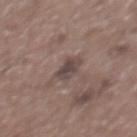Recorded during total-body skin imaging; not selected for excision or biopsy.
On the upper back.
Imaged with white-light lighting.
A male patient aged approximately 60.
A 15 mm close-up tile from a total-body photography series done for melanoma screening.
The lesion's longest dimension is about 2.5 mm.